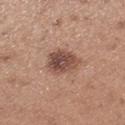Captured during whole-body skin photography for melanoma surveillance; the lesion was not biopsied.
A region of skin cropped from a whole-body photographic capture, roughly 15 mm wide.
The lesion is on the right lower leg.
Imaged with white-light lighting.
The lesion's longest dimension is about 4.5 mm.
A female patient roughly 30 years of age.